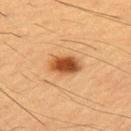notes: no biopsy performed (imaged during a skin exam) | patient: male, in their mid-50s | site: the right upper arm | image source: 15 mm crop, total-body photography | diameter: ~3.5 mm (longest diameter).